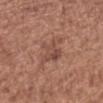The tile uses white-light illumination. The recorded lesion diameter is about 5 mm. From the left forearm. The patient is a female aged 48 to 52. This image is a 15 mm lesion crop taken from a total-body photograph.